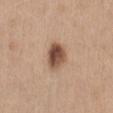Clinical impression:
The lesion was photographed on a routine skin check and not biopsied; there is no pathology result.
Clinical summary:
A female subject aged approximately 45. A close-up tile cropped from a whole-body skin photograph, about 15 mm across. Located on the abdomen.